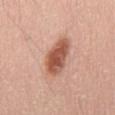  biopsy_status: not biopsied; imaged during a skin examination
  site: mid back
  patient:
    sex: male
    age_approx: 60
  image:
    source: total-body photography crop
    field_of_view_mm: 15